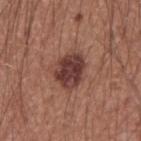biopsy_status: not biopsied; imaged during a skin examination
patient:
  sex: male
  age_approx: 60
lesion_size:
  long_diameter_mm_approx: 4.0
site: right forearm
lighting: white-light
image:
  source: total-body photography crop
  field_of_view_mm: 15